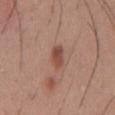Q: Is there a histopathology result?
A: no biopsy performed (imaged during a skin exam)